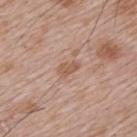Q: Was a biopsy performed?
A: imaged on a skin check; not biopsied
Q: How was this image acquired?
A: ~15 mm crop, total-body skin-cancer survey
Q: What is the anatomic site?
A: the upper back
Q: What did automated image analysis measure?
A: a footprint of about 3.5 mm², a shape eccentricity near 0.8, and two-axis asymmetry of about 0.35
Q: What are the patient's age and sex?
A: male, roughly 65 years of age
Q: How large is the lesion?
A: about 2.5 mm
Q: How was the tile lit?
A: white-light